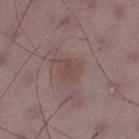| key | value |
|---|---|
| biopsy status | catalogued during a skin exam; not biopsied |
| tile lighting | white-light illumination |
| site | the leg |
| image-analysis metrics | a classifier nevus-likeness of about 15/100 and a lesion-detection confidence of about 100/100 |
| subject | male, aged 48 to 52 |
| imaging modality | ~15 mm tile from a whole-body skin photo |